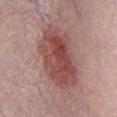biopsy status = total-body-photography surveillance lesion; no biopsy
lighting = white-light illumination
automated metrics = an area of roughly 31 mm²; a lesion–skin lightness drop of about 12; a classifier nevus-likeness of about 85/100
patient = male, approximately 30 years of age
image source = ~15 mm crop, total-body skin-cancer survey